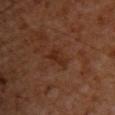workup: imaged on a skin check; not biopsied
subject: male, aged approximately 60
lesion diameter: ~3 mm (longest diameter)
body site: the upper back
tile lighting: cross-polarized
image source: 15 mm crop, total-body photography